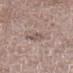{
  "biopsy_status": "not biopsied; imaged during a skin examination",
  "lighting": "white-light",
  "site": "left lower leg",
  "image": {
    "source": "total-body photography crop",
    "field_of_view_mm": 15
  },
  "patient": {
    "sex": "male",
    "age_approx": 50
  }
}